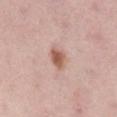Assessment:
Part of a total-body skin-imaging series; this lesion was reviewed on a skin check and was not flagged for biopsy.
Image and clinical context:
This is a white-light tile. A roughly 15 mm field-of-view crop from a total-body skin photograph. The subject is a female roughly 45 years of age. The lesion is located on the right lower leg. Longest diameter approximately 3 mm. Automated image analysis of the tile measured a lesion color around L≈57 a*≈22 b*≈28 in CIELAB. The analysis additionally found a classifier nevus-likeness of about 95/100 and a lesion-detection confidence of about 100/100.